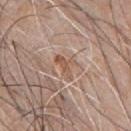workup: catalogued during a skin exam; not biopsied | anatomic site: the chest | lesion diameter: about 3.5 mm | acquisition: total-body-photography crop, ~15 mm field of view | patient: male, aged 43–47 | tile lighting: white-light.